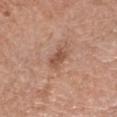Impression:
Part of a total-body skin-imaging series; this lesion was reviewed on a skin check and was not flagged for biopsy.
Context:
A male patient, in their mid- to late 60s. Cropped from a whole-body photographic skin survey; the tile spans about 15 mm. The tile uses white-light illumination. The total-body-photography lesion software estimated a lesion color around L≈52 a*≈22 b*≈29 in CIELAB, roughly 10 lightness units darker than nearby skin, and a normalized lesion–skin contrast near 7. The software also gave a color-variation rating of about 2/10. Approximately 2.5 mm at its widest. Located on the head or neck.